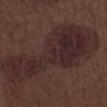Clinical impression: The lesion was tiled from a total-body skin photograph and was not biopsied. Background: Cropped from a total-body skin-imaging series; the visible field is about 15 mm. Captured under white-light illumination. The lesion is located on the right lower leg. Automated image analysis of the tile measured a footprint of about 55 mm², an outline eccentricity of about 0.9 (0 = round, 1 = elongated), and two-axis asymmetry of about 0.45. About 13 mm across. A male patient approximately 70 years of age.